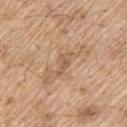Q: Was a biopsy performed?
A: no biopsy performed (imaged during a skin exam)
Q: Lesion location?
A: the upper back
Q: Who is the patient?
A: male, about 70 years old
Q: What is the imaging modality?
A: total-body-photography crop, ~15 mm field of view